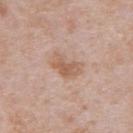{"biopsy_status": "not biopsied; imaged during a skin examination", "site": "abdomen", "patient": {"sex": "male", "age_approx": 65}, "image": {"source": "total-body photography crop", "field_of_view_mm": 15}}